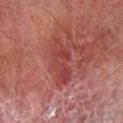The lesion is on the leg. A close-up tile cropped from a whole-body skin photograph, about 15 mm across. The tile uses cross-polarized illumination. A male subject, about 70 years old. About 5.5 mm across.